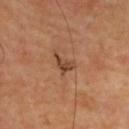notes: imaged on a skin check; not biopsied | diameter: ~3 mm (longest diameter) | TBP lesion metrics: an area of roughly 4 mm², an outline eccentricity of about 0.75 (0 = round, 1 = elongated), and a symmetry-axis asymmetry near 0.5; a border-irregularity rating of about 4.5/10 and a within-lesion color-variation index near 2.5/10 | body site: the upper back | imaging modality: 15 mm crop, total-body photography | patient: female, in their mid-40s | tile lighting: cross-polarized.